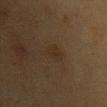| key | value |
|---|---|
| biopsy status | no biopsy performed (imaged during a skin exam) |
| subject | female, aged around 40 |
| image source | total-body-photography crop, ~15 mm field of view |
| automated metrics | a nevus-likeness score of about 0/100 |
| anatomic site | the chest |
| illumination | cross-polarized |
| size | about 2.5 mm |